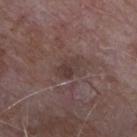Case summary:
• subject: male, roughly 65 years of age
• acquisition: ~15 mm crop, total-body skin-cancer survey
• location: the chest
• lighting: white-light illumination